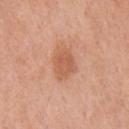follow-up — total-body-photography surveillance lesion; no biopsy
diameter — ~3.5 mm (longest diameter)
subject — male, aged approximately 70
location — the chest
imaging modality — 15 mm crop, total-body photography